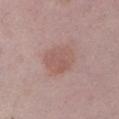Case summary:
- workup: imaged on a skin check; not biopsied
- imaging modality: 15 mm crop, total-body photography
- automated lesion analysis: a lesion area of about 12 mm², a shape eccentricity near 0.6, and two-axis asymmetry of about 0.1; about 7 CIELAB-L* units darker than the surrounding skin and a normalized lesion–skin contrast near 5.5; an automated nevus-likeness rating near 55 out of 100 and a lesion-detection confidence of about 100/100
- diameter: ≈4.5 mm
- location: the left upper arm
- illumination: white-light
- subject: male, aged around 50